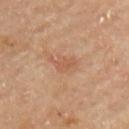Q: What is the imaging modality?
A: ~15 mm crop, total-body skin-cancer survey
Q: Lesion location?
A: the chest
Q: Illumination type?
A: cross-polarized
Q: What did automated image analysis measure?
A: a footprint of about 4 mm² and an outline eccentricity of about 0.8 (0 = round, 1 = elongated); border irregularity of about 5.5 on a 0–10 scale, a color-variation rating of about 1.5/10, and radial color variation of about 0.5
Q: Who is the patient?
A: male, aged approximately 65